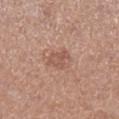Recorded during total-body skin imaging; not selected for excision or biopsy. The subject is a female approximately 40 years of age. A lesion tile, about 15 mm wide, cut from a 3D total-body photograph. The lesion is on the right lower leg.